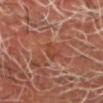No biopsy was performed on this lesion — it was imaged during a full skin examination and was not determined to be concerning. Imaged with cross-polarized lighting. Located on the head or neck. Longest diameter approximately 2.5 mm. A male subject in their 70s. A region of skin cropped from a whole-body photographic capture, roughly 15 mm wide.